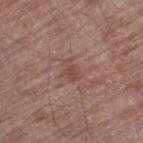| feature | finding |
|---|---|
| workup | no biopsy performed (imaged during a skin exam) |
| image source | ~15 mm crop, total-body skin-cancer survey |
| subject | male, approximately 70 years of age |
| location | the left thigh |
| diameter | about 2.5 mm |